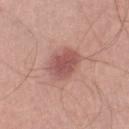Located on the right lower leg. The subject is a male in their mid- to late 50s. The tile uses white-light illumination. The recorded lesion diameter is about 4 mm. The lesion-visualizer software estimated a lesion area of about 10 mm² and a shape-asymmetry score of about 0.1 (0 = symmetric). A 15 mm crop from a total-body photograph taken for skin-cancer surveillance.